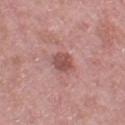Q: Was this lesion biopsied?
A: total-body-photography surveillance lesion; no biopsy
Q: How large is the lesion?
A: about 2.5 mm
Q: What did automated image analysis measure?
A: an area of roughly 4.5 mm²; a lesion color around L≈50 a*≈25 b*≈22 in CIELAB and a lesion–skin lightness drop of about 11; border irregularity of about 1.5 on a 0–10 scale and radial color variation of about 1; a classifier nevus-likeness of about 10/100 and lesion-presence confidence of about 100/100
Q: What is the anatomic site?
A: the leg
Q: Illumination type?
A: white-light
Q: What kind of image is this?
A: total-body-photography crop, ~15 mm field of view
Q: Patient demographics?
A: female, aged 38 to 42